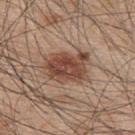The lesion was tiled from a total-body skin photograph and was not biopsied. A male subject, aged around 45. This is a white-light tile. The lesion is located on the upper back. A lesion tile, about 15 mm wide, cut from a 3D total-body photograph. The lesion's longest dimension is about 5.5 mm.